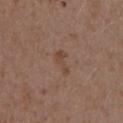{
  "biopsy_status": "not biopsied; imaged during a skin examination",
  "image": {
    "source": "total-body photography crop",
    "field_of_view_mm": 15
  },
  "lesion_size": {
    "long_diameter_mm_approx": 3.0
  },
  "automated_metrics": {
    "cielab_L": 44,
    "cielab_a": 18,
    "cielab_b": 26,
    "vs_skin_darker_L": 6.0,
    "vs_skin_contrast_norm": 5.5,
    "lesion_detection_confidence_0_100": 100
  },
  "patient": {
    "sex": "male",
    "age_approx": 70
  },
  "site": "left upper arm"
}